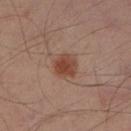Part of a total-body skin-imaging series; this lesion was reviewed on a skin check and was not flagged for biopsy.
Cropped from a whole-body photographic skin survey; the tile spans about 15 mm.
The lesion is located on the left lower leg.
Automated tile analysis of the lesion measured an area of roughly 6.5 mm². And it measured a lesion color around L≈43 a*≈22 b*≈28 in CIELAB, roughly 10 lightness units darker than nearby skin, and a normalized border contrast of about 8.5. The analysis additionally found a border-irregularity index near 1.5/10, a within-lesion color-variation index near 3.5/10, and radial color variation of about 1. The analysis additionally found a nevus-likeness score of about 100/100 and a lesion-detection confidence of about 100/100.
This is a cross-polarized tile.
The subject is aged 53 to 57.